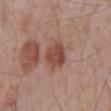Findings:
– biopsy status: total-body-photography surveillance lesion; no biopsy
– body site: the abdomen
– TBP lesion metrics: a footprint of about 8.5 mm², a shape eccentricity near 0.5, and a shape-asymmetry score of about 0.15 (0 = symmetric); an average lesion color of about L≈47 a*≈23 b*≈27 (CIELAB) and about 11 CIELAB-L* units darker than the surrounding skin; a nevus-likeness score of about 30/100
– patient: male, aged 68–72
– lesion diameter: about 3.5 mm
– lighting: white-light
– image source: total-body-photography crop, ~15 mm field of view